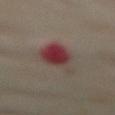Imaged during a routine full-body skin examination; the lesion was not biopsied and no histopathology is available. The subject is a female approximately 55 years of age. The lesion is on the abdomen. About 6 mm across. The tile uses cross-polarized illumination. A 15 mm close-up extracted from a 3D total-body photography capture.